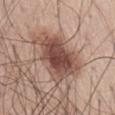biopsy status: imaged on a skin check; not biopsied
lighting: white-light
site: the abdomen
image: ~15 mm crop, total-body skin-cancer survey
lesion diameter: about 7.5 mm
automated lesion analysis: an average lesion color of about L≈49 a*≈20 b*≈24 (CIELAB) and a lesion–skin lightness drop of about 14; a border-irregularity rating of about 4/10, internal color variation of about 6 on a 0–10 scale, and peripheral color asymmetry of about 1.5
patient: male, aged approximately 40